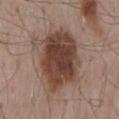biopsy status = imaged on a skin check; not biopsied | patient = male, aged around 55 | imaging modality = 15 mm crop, total-body photography | location = the mid back | automated metrics = an eccentricity of roughly 0.7 and a symmetry-axis asymmetry near 0.25; a color-variation rating of about 5.5/10 and radial color variation of about 1.5; an automated nevus-likeness rating near 95 out of 100 and lesion-presence confidence of about 100/100.